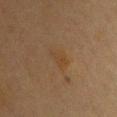Q: Is there a histopathology result?
A: total-body-photography surveillance lesion; no biopsy
Q: Patient demographics?
A: female, approximately 40 years of age
Q: Lesion location?
A: the front of the torso
Q: How was the tile lit?
A: cross-polarized
Q: What is the imaging modality?
A: 15 mm crop, total-body photography